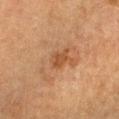{
  "biopsy_status": "not biopsied; imaged during a skin examination",
  "patient": {
    "sex": "female",
    "age_approx": 55
  },
  "lesion_size": {
    "long_diameter_mm_approx": 3.0
  },
  "lighting": "cross-polarized",
  "image": {
    "source": "total-body photography crop",
    "field_of_view_mm": 15
  },
  "site": "right forearm",
  "automated_metrics": {
    "border_irregularity_0_10": 3.5,
    "color_variation_0_10": 1.5,
    "peripheral_color_asymmetry": 0.5,
    "nevus_likeness_0_100": 0,
    "lesion_detection_confidence_0_100": 100
  }
}